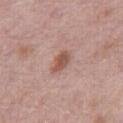Q: Is there a histopathology result?
A: total-body-photography surveillance lesion; no biopsy
Q: Who is the patient?
A: female, aged approximately 65
Q: What kind of image is this?
A: 15 mm crop, total-body photography
Q: Lesion location?
A: the right thigh
Q: How large is the lesion?
A: ≈3 mm